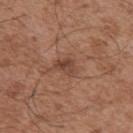Recorded during total-body skin imaging; not selected for excision or biopsy.
This is a white-light tile.
Longest diameter approximately 3 mm.
A 15 mm crop from a total-body photograph taken for skin-cancer surveillance.
A male patient, roughly 50 years of age.
The lesion is on the left upper arm.